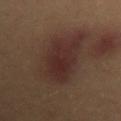The lesion was tiled from a total-body skin photograph and was not biopsied. Automated image analysis of the tile measured an area of roughly 29 mm², a shape eccentricity near 0.85, and a symmetry-axis asymmetry near 0.2. The subject is a female aged 38 to 42. A 15 mm close-up extracted from a 3D total-body photography capture. Located on the back. Imaged with cross-polarized lighting.